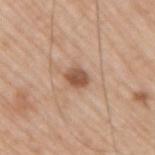<tbp_lesion>
<biopsy_status>not biopsied; imaged during a skin examination</biopsy_status>
<lesion_size>
  <long_diameter_mm_approx>2.5</long_diameter_mm_approx>
</lesion_size>
<patient>
  <sex>male</sex>
  <age_approx>65</age_approx>
</patient>
<image>
  <source>total-body photography crop</source>
  <field_of_view_mm>15</field_of_view_mm>
</image>
<site>right upper arm</site>
<lighting>white-light</lighting>
</tbp_lesion>